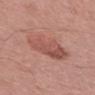notes: total-body-photography surveillance lesion; no biopsy | automated metrics: an outline eccentricity of about 0.65 (0 = round, 1 = elongated) and a shape-asymmetry score of about 0.15 (0 = symmetric); border irregularity of about 2 on a 0–10 scale, a color-variation rating of about 5/10, and peripheral color asymmetry of about 2; a classifier nevus-likeness of about 25/100 and lesion-presence confidence of about 100/100 | body site: the left forearm | size: ~5.5 mm (longest diameter) | illumination: white-light illumination | patient: female, approximately 65 years of age | image source: total-body-photography crop, ~15 mm field of view.